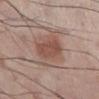Assessment: Part of a total-body skin-imaging series; this lesion was reviewed on a skin check and was not flagged for biopsy. Acquisition and patient details: The lesion is located on the leg. A male subject in their 60s. The lesion-visualizer software estimated an area of roughly 18 mm², an outline eccentricity of about 0.75 (0 = round, 1 = elongated), and a symmetry-axis asymmetry near 0.3. The analysis additionally found a mean CIELAB color near L≈52 a*≈19 b*≈25, a lesion–skin lightness drop of about 9, and a lesion-to-skin contrast of about 6.5 (normalized; higher = more distinct). It also reported a color-variation rating of about 4.5/10. And it measured a nevus-likeness score of about 100/100 and a lesion-detection confidence of about 100/100. Cropped from a total-body skin-imaging series; the visible field is about 15 mm. The recorded lesion diameter is about 6.5 mm.